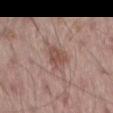Impression:
The lesion was photographed on a routine skin check and not biopsied; there is no pathology result.
Clinical summary:
A 15 mm close-up extracted from a 3D total-body photography capture. The lesion is on the left thigh. About 3 mm across. Captured under white-light illumination. A male subject approximately 55 years of age. The total-body-photography lesion software estimated a mean CIELAB color near L≈50 a*≈20 b*≈24, roughly 9 lightness units darker than nearby skin, and a normalized border contrast of about 7. The analysis additionally found a border-irregularity index near 3/10, internal color variation of about 2.5 on a 0–10 scale, and radial color variation of about 1. The analysis additionally found a nevus-likeness score of about 80/100 and lesion-presence confidence of about 100/100.